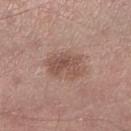Clinical impression:
The lesion was photographed on a routine skin check and not biopsied; there is no pathology result.
Clinical summary:
The lesion-visualizer software estimated an eccentricity of roughly 0.7. It also reported about 9 CIELAB-L* units darker than the surrounding skin and a normalized border contrast of about 6.5. And it measured a classifier nevus-likeness of about 10/100. Cropped from a total-body skin-imaging series; the visible field is about 15 mm. Imaged with white-light lighting. A male patient aged approximately 55. The lesion is on the right lower leg.